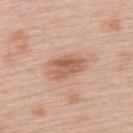| feature | finding |
|---|---|
| notes | no biopsy performed (imaged during a skin exam) |
| tile lighting | white-light illumination |
| site | the upper back |
| patient | female, in their mid-60s |
| lesion diameter | ≈5 mm |
| image-analysis metrics | an area of roughly 9.5 mm², an eccentricity of roughly 0.85, and a symmetry-axis asymmetry near 0.2; a lesion color around L≈60 a*≈22 b*≈31 in CIELAB, a lesion–skin lightness drop of about 11, and a normalized lesion–skin contrast near 7.5; a detector confidence of about 100 out of 100 that the crop contains a lesion |
| image | total-body-photography crop, ~15 mm field of view |